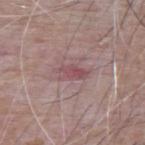| feature | finding |
|---|---|
| notes | total-body-photography surveillance lesion; no biopsy |
| imaging modality | total-body-photography crop, ~15 mm field of view |
| patient | male, aged approximately 65 |
| illumination | white-light |
| site | the upper back |
| diameter | about 3.5 mm |